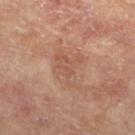| field | value |
|---|---|
| workup | no biopsy performed (imaged during a skin exam) |
| anatomic site | the right lower leg |
| patient | female, aged 73–77 |
| diameter | about 4 mm |
| image-analysis metrics | a footprint of about 8 mm² and a shape eccentricity near 0.7; an average lesion color of about L≈54 a*≈22 b*≈29 (CIELAB), roughly 6 lightness units darker than nearby skin, and a lesion-to-skin contrast of about 4.5 (normalized; higher = more distinct); a border-irregularity index near 5.5/10 and internal color variation of about 2.5 on a 0–10 scale; a nevus-likeness score of about 0/100 and lesion-presence confidence of about 100/100 |
| image source | 15 mm crop, total-body photography |